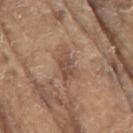The lesion was tiled from a total-body skin photograph and was not biopsied. A male subject in their 80s. From the right upper arm. Longest diameter approximately 3.5 mm. A roughly 15 mm field-of-view crop from a total-body skin photograph.